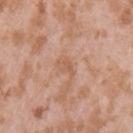Impression: Recorded during total-body skin imaging; not selected for excision or biopsy.